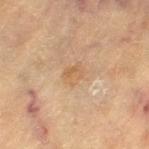{"biopsy_status": "not biopsied; imaged during a skin examination", "site": "left thigh", "automated_metrics": {"nevus_likeness_0_100": 0, "lesion_detection_confidence_0_100": 100}, "patient": {"sex": "female", "age_approx": 80}, "image": {"source": "total-body photography crop", "field_of_view_mm": 15}, "lesion_size": {"long_diameter_mm_approx": 2.5}}